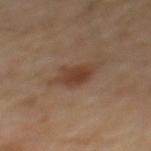Imaged during a routine full-body skin examination; the lesion was not biopsied and no histopathology is available.